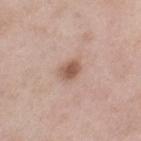Q: Is there a histopathology result?
A: total-body-photography surveillance lesion; no biopsy
Q: How was this image acquired?
A: ~15 mm crop, total-body skin-cancer survey
Q: Lesion size?
A: ≈2.5 mm
Q: How was the tile lit?
A: white-light
Q: Patient demographics?
A: female, aged approximately 55
Q: Where on the body is the lesion?
A: the left thigh
Q: Automated lesion metrics?
A: border irregularity of about 2 on a 0–10 scale and peripheral color asymmetry of about 1; a nevus-likeness score of about 85/100 and a lesion-detection confidence of about 100/100